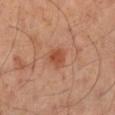Clinical impression:
Recorded during total-body skin imaging; not selected for excision or biopsy.
Background:
On the leg. A region of skin cropped from a whole-body photographic capture, roughly 15 mm wide. The tile uses cross-polarized illumination. A male subject aged 53 to 57. The recorded lesion diameter is about 2.5 mm.